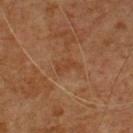Assessment: Part of a total-body skin-imaging series; this lesion was reviewed on a skin check and was not flagged for biopsy. Context: The lesion is located on the front of the torso. A region of skin cropped from a whole-body photographic capture, roughly 15 mm wide. A male subject aged around 55. Imaged with cross-polarized lighting.